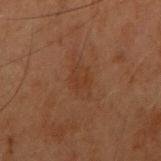Part of a total-body skin-imaging series; this lesion was reviewed on a skin check and was not flagged for biopsy.
The lesion's longest dimension is about 3.5 mm.
On the arm.
A male patient aged around 60.
Captured under cross-polarized illumination.
Cropped from a whole-body photographic skin survey; the tile spans about 15 mm.